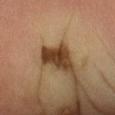The lesion was tiled from a total-body skin photograph and was not biopsied.
A close-up tile cropped from a whole-body skin photograph, about 15 mm across.
The subject is a male approximately 65 years of age.